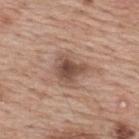workup: imaged on a skin check; not biopsied | anatomic site: the back | acquisition: ~15 mm crop, total-body skin-cancer survey | patient: male, roughly 70 years of age | lighting: white-light illumination.